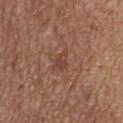No biopsy was performed on this lesion — it was imaged during a full skin examination and was not determined to be concerning. From the upper back. Cropped from a whole-body photographic skin survey; the tile spans about 15 mm. The patient is a female aged 53 to 57.